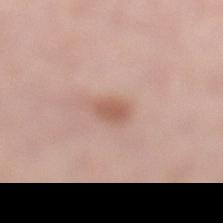{
  "lighting": "white-light",
  "lesion_size": {
    "long_diameter_mm_approx": 2.5
  },
  "patient": {
    "sex": "female",
    "age_approx": 50
  },
  "site": "left lower leg",
  "automated_metrics": {
    "cielab_L": 58,
    "cielab_a": 21,
    "cielab_b": 28,
    "vs_skin_contrast_norm": 7.0,
    "nevus_likeness_0_100": 80,
    "lesion_detection_confidence_0_100": 100
  },
  "image": {
    "source": "total-body photography crop",
    "field_of_view_mm": 15
  }
}